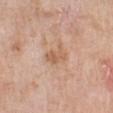{"biopsy_status": "not biopsied; imaged during a skin examination", "patient": {"sex": "female", "age_approx": 70}, "site": "left lower leg", "image": {"source": "total-body photography crop", "field_of_view_mm": 15}}